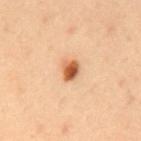follow-up = no biopsy performed (imaged during a skin exam) | site = the mid back | imaging modality = ~15 mm tile from a whole-body skin photo | subject = male, aged 33 to 37.